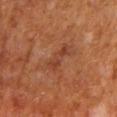| key | value |
|---|---|
| biopsy status | total-body-photography surveillance lesion; no biopsy |
| image source | total-body-photography crop, ~15 mm field of view |
| site | the back |
| lesion diameter | ~3.5 mm (longest diameter) |
| tile lighting | cross-polarized |
| image-analysis metrics | a lesion area of about 5 mm², an outline eccentricity of about 0.95 (0 = round, 1 = elongated), and two-axis asymmetry of about 0.35; a lesion color around L≈40 a*≈25 b*≈31 in CIELAB and a normalized lesion–skin contrast near 5.5; border irregularity of about 4.5 on a 0–10 scale and peripheral color asymmetry of about 0; a classifier nevus-likeness of about 0/100 and lesion-presence confidence of about 100/100 |
| patient | male, about 65 years old |